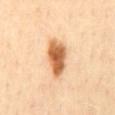Part of a total-body skin-imaging series; this lesion was reviewed on a skin check and was not flagged for biopsy. The subject is a female about 45 years old. On the mid back. A 15 mm crop from a total-body photograph taken for skin-cancer surveillance.